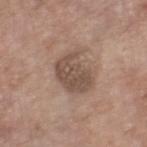| feature | finding |
|---|---|
| follow-up | imaged on a skin check; not biopsied |
| body site | the right lower leg |
| subject | male, aged around 80 |
| acquisition | ~15 mm crop, total-body skin-cancer survey |
| automated metrics | a mean CIELAB color near L≈50 a*≈16 b*≈25, roughly 10 lightness units darker than nearby skin, and a normalized lesion–skin contrast near 7.5; border irregularity of about 3 on a 0–10 scale and a peripheral color-asymmetry measure near 1; a classifier nevus-likeness of about 20/100 and a detector confidence of about 100 out of 100 that the crop contains a lesion |